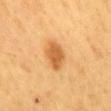– workup: total-body-photography surveillance lesion; no biopsy
– image: total-body-photography crop, ~15 mm field of view
– site: the mid back
– subject: male, about 60 years old
– image-analysis metrics: a mean CIELAB color near L≈61 a*≈25 b*≈47, roughly 12 lightness units darker than nearby skin, and a lesion-to-skin contrast of about 8 (normalized; higher = more distinct)
– illumination: cross-polarized illumination
– diameter: about 3.5 mm